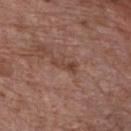The lesion was tiled from a total-body skin photograph and was not biopsied.
The lesion is located on the front of the torso.
Cropped from a total-body skin-imaging series; the visible field is about 15 mm.
The tile uses white-light illumination.
A male patient roughly 60 years of age.
The lesion's longest dimension is about 3.5 mm.